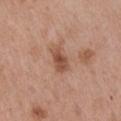Clinical impression: Recorded during total-body skin imaging; not selected for excision or biopsy. Acquisition and patient details: A 15 mm close-up extracted from a 3D total-body photography capture. The tile uses white-light illumination. The lesion-visualizer software estimated an area of roughly 5 mm² and two-axis asymmetry of about 0.2. The analysis additionally found a lesion color around L≈50 a*≈23 b*≈30 in CIELAB and a lesion–skin lightness drop of about 11. It also reported a border-irregularity index near 2.5/10, a within-lesion color-variation index near 3/10, and peripheral color asymmetry of about 1. From the chest. About 3 mm across. The subject is a female in their mid- to late 60s.